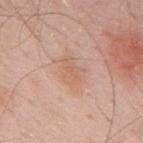This lesion was catalogued during total-body skin photography and was not selected for biopsy. From the mid back. An algorithmic analysis of the crop reported a lesion area of about 6 mm², a shape eccentricity near 0.7, and a shape-asymmetry score of about 0.25 (0 = symmetric). The analysis additionally found about 6 CIELAB-L* units darker than the surrounding skin and a normalized lesion–skin contrast near 4.5. The analysis additionally found a classifier nevus-likeness of about 0/100 and a detector confidence of about 100 out of 100 that the crop contains a lesion. Longest diameter approximately 3 mm. A male patient, in their mid- to late 50s. Imaged with white-light lighting. This image is a 15 mm lesion crop taken from a total-body photograph.